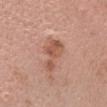  biopsy_status: not biopsied; imaged during a skin examination
  automated_metrics:
    cielab_L: 55
    cielab_a: 23
    cielab_b: 30
    border_irregularity_0_10: 6.5
    color_variation_0_10: 3.5
    peripheral_color_asymmetry: 1.0
    nevus_likeness_0_100: 5
    lesion_detection_confidence_0_100: 100
  image:
    source: total-body photography crop
    field_of_view_mm: 15
  lighting: white-light
  site: head or neck
  patient:
    sex: female
    age_approx: 20